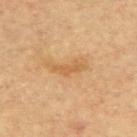Clinical impression: Imaged during a routine full-body skin examination; the lesion was not biopsied and no histopathology is available. Acquisition and patient details: The patient is a female roughly 80 years of age. Captured under cross-polarized illumination. Approximately 3.5 mm at its widest. An algorithmic analysis of the crop reported two-axis asymmetry of about 0.45. It also reported a border-irregularity rating of about 5/10 and a peripheral color-asymmetry measure near 0. The lesion is on the upper back. A 15 mm close-up tile from a total-body photography series done for melanoma screening.